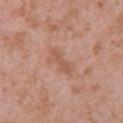Recorded during total-body skin imaging; not selected for excision or biopsy.
From the upper back.
Cropped from a total-body skin-imaging series; the visible field is about 15 mm.
The subject is a female roughly 40 years of age.
This is a white-light tile.